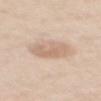Impression: Recorded during total-body skin imaging; not selected for excision or biopsy. Acquisition and patient details: The lesion is located on the mid back. A male patient, in their mid- to late 60s. A roughly 15 mm field-of-view crop from a total-body skin photograph.